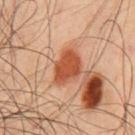Captured during whole-body skin photography for melanoma surveillance; the lesion was not biopsied. The recorded lesion diameter is about 4.5 mm. The lesion is on the chest. The subject is a male about 45 years old. A region of skin cropped from a whole-body photographic capture, roughly 15 mm wide. The tile uses cross-polarized illumination.